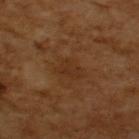  biopsy_status: not biopsied; imaged during a skin examination
  image:
    source: total-body photography crop
    field_of_view_mm: 15
  automated_metrics:
    cielab_L: 30
    cielab_a: 19
    cielab_b: 31
    vs_skin_contrast_norm: 5.0
  patient:
    sex: male
    age_approx: 65
  lighting: cross-polarized